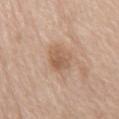Impression: The lesion was tiled from a total-body skin photograph and was not biopsied. Clinical summary: A female patient aged 73–77. Located on the mid back. A region of skin cropped from a whole-body photographic capture, roughly 15 mm wide. Automated image analysis of the tile measured a mean CIELAB color near L≈58 a*≈18 b*≈31 and about 9 CIELAB-L* units darker than the surrounding skin. And it measured a border-irregularity index near 2/10, internal color variation of about 3.5 on a 0–10 scale, and a peripheral color-asymmetry measure near 1. And it measured a classifier nevus-likeness of about 0/100 and a detector confidence of about 100 out of 100 that the crop contains a lesion. Imaged with white-light lighting.